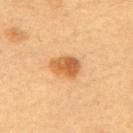<tbp_lesion>
  <biopsy_status>not biopsied; imaged during a skin examination</biopsy_status>
</tbp_lesion>